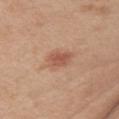follow-up: total-body-photography surveillance lesion; no biopsy
patient: female, aged approximately 40
acquisition: ~15 mm tile from a whole-body skin photo
anatomic site: the arm